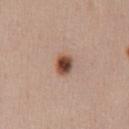- site: the mid back
- acquisition: total-body-photography crop, ~15 mm field of view
- patient: female, about 50 years old